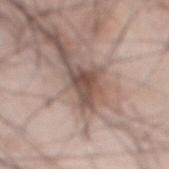No biopsy was performed on this lesion — it was imaged during a full skin examination and was not determined to be concerning.
A male subject, in their 60s.
A close-up tile cropped from a whole-body skin photograph, about 15 mm across.
Longest diameter approximately 4 mm.
The lesion is on the front of the torso.